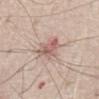Acquisition and patient details: The tile uses white-light illumination. Longest diameter approximately 3.5 mm. Located on the front of the torso. The subject is a male roughly 70 years of age. A close-up tile cropped from a whole-body skin photograph, about 15 mm across.